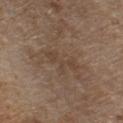{"image": {"source": "total-body photography crop", "field_of_view_mm": 15}, "site": "chest", "lesion_size": {"long_diameter_mm_approx": 5.0}, "patient": {"sex": "male", "age_approx": 70}, "lighting": "white-light"}